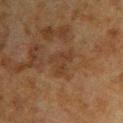Recorded during total-body skin imaging; not selected for excision or biopsy. Cropped from a total-body skin-imaging series; the visible field is about 15 mm. The lesion is on the arm. A male patient, in their 60s. Measured at roughly 3 mm in maximum diameter. Captured under cross-polarized illumination.